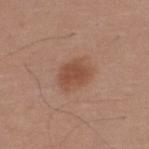The lesion is located on the upper back. Cropped from a whole-body photographic skin survey; the tile spans about 15 mm. The total-body-photography lesion software estimated a lesion–skin lightness drop of about 9 and a normalized border contrast of about 7.5. The software also gave a within-lesion color-variation index near 2/10 and peripheral color asymmetry of about 0.5. The analysis additionally found a nevus-likeness score of about 90/100 and a detector confidence of about 100 out of 100 that the crop contains a lesion. A male patient roughly 40 years of age. Captured under white-light illumination. Approximately 3.5 mm at its widest.